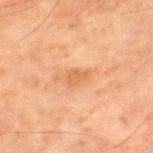subject = male, aged around 80; lesion diameter = ~2.5 mm (longest diameter); location = the right thigh; imaging modality = 15 mm crop, total-body photography; tile lighting = cross-polarized illumination.